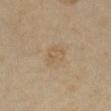Findings:
• workup · total-body-photography surveillance lesion; no biopsy
• lesion size · ≈3 mm
• image source · 15 mm crop, total-body photography
• patient · female, approximately 35 years of age
• image-analysis metrics · an area of roughly 5 mm², a shape eccentricity near 0.7, and a symmetry-axis asymmetry near 0.3; a nevus-likeness score of about 0/100
• location · the front of the torso
• illumination · cross-polarized illumination